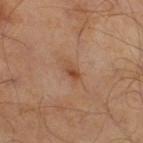Assessment:
The lesion was tiled from a total-body skin photograph and was not biopsied.
Background:
The recorded lesion diameter is about 2.5 mm. A lesion tile, about 15 mm wide, cut from a 3D total-body photograph. The total-body-photography lesion software estimated a within-lesion color-variation index near 4/10 and radial color variation of about 1. And it measured an automated nevus-likeness rating near 20 out of 100. A male subject, aged 58–62. Located on the leg. Imaged with cross-polarized lighting.